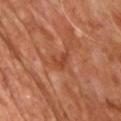Q: Is there a histopathology result?
A: imaged on a skin check; not biopsied
Q: How was the tile lit?
A: cross-polarized illumination
Q: Patient demographics?
A: male, in their mid-60s
Q: What is the imaging modality?
A: ~15 mm tile from a whole-body skin photo
Q: What did automated image analysis measure?
A: a border-irregularity index near 5/10, internal color variation of about 1 on a 0–10 scale, and radial color variation of about 0.5; an automated nevus-likeness rating near 0 out of 100
Q: Lesion size?
A: ≈2.5 mm